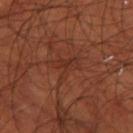<lesion>
<biopsy_status>not biopsied; imaged during a skin examination</biopsy_status>
<site>left thigh</site>
<lighting>cross-polarized</lighting>
<patient>
  <sex>male</sex>
  <age_approx>70</age_approx>
</patient>
<lesion_size>
  <long_diameter_mm_approx>3.5</long_diameter_mm_approx>
</lesion_size>
<image>
  <source>total-body photography crop</source>
  <field_of_view_mm>15</field_of_view_mm>
</image>
</lesion>